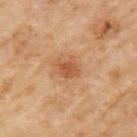follow-up: no biopsy performed (imaged during a skin exam) | subject: female, roughly 60 years of age | acquisition: total-body-photography crop, ~15 mm field of view | lesion size: ≈3 mm | body site: the left upper arm | illumination: cross-polarized | image-analysis metrics: a footprint of about 5 mm², an outline eccentricity of about 0.55 (0 = round, 1 = elongated), and a shape-asymmetry score of about 0.2 (0 = symmetric); a lesion color around L≈55 a*≈23 b*≈38 in CIELAB, about 9 CIELAB-L* units darker than the surrounding skin, and a normalized border contrast of about 7; radial color variation of about 1; a classifier nevus-likeness of about 50/100 and lesion-presence confidence of about 100/100.